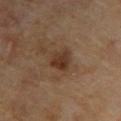Captured during whole-body skin photography for melanoma surveillance; the lesion was not biopsied.
The lesion is on the right upper arm.
A male patient approximately 65 years of age.
Imaged with cross-polarized lighting.
Longest diameter approximately 3.5 mm.
A 15 mm crop from a total-body photograph taken for skin-cancer surveillance.